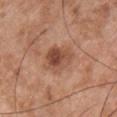follow-up — catalogued during a skin exam; not biopsied
acquisition — 15 mm crop, total-body photography
size — ~3.5 mm (longest diameter)
tile lighting — white-light illumination
site — the chest
subject — male, aged 48 to 52